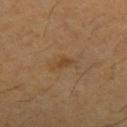follow-up: no biopsy performed (imaged during a skin exam); patient: male, roughly 60 years of age; automated metrics: an eccentricity of roughly 0.8 and a symmetry-axis asymmetry near 0.3; body site: the right thigh; lighting: cross-polarized; image source: 15 mm crop, total-body photography; diameter: about 1.5 mm.